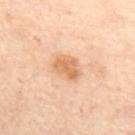Assessment:
Part of a total-body skin-imaging series; this lesion was reviewed on a skin check and was not flagged for biopsy.
Background:
A female subject aged around 55. The lesion's longest dimension is about 3 mm. From the chest. Captured under cross-polarized illumination. Automated tile analysis of the lesion measured a border-irregularity index near 2/10, a within-lesion color-variation index near 2/10, and radial color variation of about 0.5. A region of skin cropped from a whole-body photographic capture, roughly 15 mm wide.